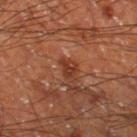Clinical impression: Part of a total-body skin-imaging series; this lesion was reviewed on a skin check and was not flagged for biopsy. Image and clinical context: The lesion is on the left thigh. A male subject, aged around 60. Cropped from a total-body skin-imaging series; the visible field is about 15 mm.